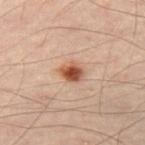This lesion was catalogued during total-body skin photography and was not selected for biopsy.
A 15 mm crop from a total-body photograph taken for skin-cancer surveillance.
On the left thigh.
The tile uses cross-polarized illumination.
The subject is a male approximately 50 years of age.